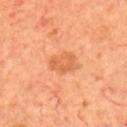Impression:
Recorded during total-body skin imaging; not selected for excision or biopsy.
Acquisition and patient details:
The total-body-photography lesion software estimated an area of roughly 7 mm² and two-axis asymmetry of about 0.25. The software also gave a border-irregularity index near 2.5/10, a color-variation rating of about 2/10, and peripheral color asymmetry of about 0.5. And it measured a classifier nevus-likeness of about 20/100. A 15 mm close-up tile from a total-body photography series done for melanoma screening. A male patient aged 68–72. On the upper back. The lesion's longest dimension is about 4 mm. This is a cross-polarized tile.